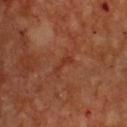Clinical impression: The lesion was photographed on a routine skin check and not biopsied; there is no pathology result. Background: The total-body-photography lesion software estimated an area of roughly 2 mm², an outline eccentricity of about 0.95 (0 = round, 1 = elongated), and a symmetry-axis asymmetry near 0.4. And it measured an average lesion color of about L≈36 a*≈28 b*≈32 (CIELAB) and a normalized lesion–skin contrast near 5.5. It also reported lesion-presence confidence of about 95/100. Imaged with cross-polarized lighting. Measured at roughly 3 mm in maximum diameter. The lesion is on the chest. A male patient aged approximately 50. A lesion tile, about 15 mm wide, cut from a 3D total-body photograph.